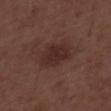<tbp_lesion>
<biopsy_status>not biopsied; imaged during a skin examination</biopsy_status>
<image>
  <source>total-body photography crop</source>
  <field_of_view_mm>15</field_of_view_mm>
</image>
<patient>
  <sex>male</sex>
  <age_approx>50</age_approx>
</patient>
</tbp_lesion>